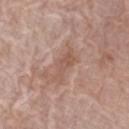Findings:
• anatomic site: the arm
• patient: female, in their mid-70s
• size: ~4 mm (longest diameter)
• illumination: white-light illumination
• acquisition: ~15 mm crop, total-body skin-cancer survey
• TBP lesion metrics: a footprint of about 5.5 mm², an eccentricity of roughly 0.9, and a symmetry-axis asymmetry near 0.4; a border-irregularity index near 5/10, a color-variation rating of about 1.5/10, and peripheral color asymmetry of about 0.5; an automated nevus-likeness rating near 0 out of 100